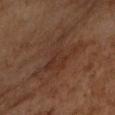Case summary:
* follow-up: catalogued during a skin exam; not biopsied
* tile lighting: cross-polarized illumination
* subject: female, roughly 60 years of age
* body site: the right upper arm
* image: total-body-photography crop, ~15 mm field of view
* diameter: ≈7.5 mm